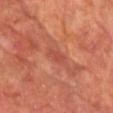Q: Is there a histopathology result?
A: catalogued during a skin exam; not biopsied
Q: Illumination type?
A: cross-polarized illumination
Q: What is the imaging modality?
A: ~15 mm crop, total-body skin-cancer survey
Q: Who is the patient?
A: male, roughly 65 years of age
Q: What is the anatomic site?
A: the front of the torso
Q: What is the lesion's diameter?
A: about 3 mm